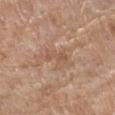Imaged during a routine full-body skin examination; the lesion was not biopsied and no histopathology is available. The tile uses white-light illumination. A female subject in their mid- to late 70s. On the left thigh. Automated image analysis of the tile measured a normalized lesion–skin contrast near 5. The recorded lesion diameter is about 3 mm. A 15 mm close-up tile from a total-body photography series done for melanoma screening.